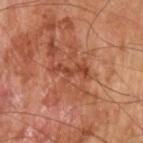workup = total-body-photography surveillance lesion; no biopsy | patient = male, in their mid-60s | tile lighting = cross-polarized | lesion size = ~4 mm (longest diameter) | acquisition = ~15 mm tile from a whole-body skin photo | TBP lesion metrics = a lesion area of about 5.5 mm² and an eccentricity of roughly 0.9 | body site = the left upper arm.